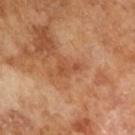Clinical impression: The lesion was tiled from a total-body skin photograph and was not biopsied. Acquisition and patient details: Cropped from a whole-body photographic skin survey; the tile spans about 15 mm. Measured at roughly 2.5 mm in maximum diameter. Imaged with cross-polarized lighting. A male patient, aged around 65.